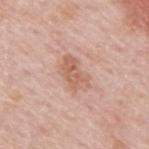Image and clinical context: Cropped from a total-body skin-imaging series; the visible field is about 15 mm. A male subject, about 60 years old. From the upper back. This is a white-light tile.